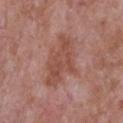workup = no biopsy performed (imaged during a skin exam)
acquisition = ~15 mm tile from a whole-body skin photo
automated lesion analysis = border irregularity of about 5.5 on a 0–10 scale and peripheral color asymmetry of about 1; a nevus-likeness score of about 0/100 and lesion-presence confidence of about 100/100
body site = the upper back
subject = male, in their mid- to late 60s